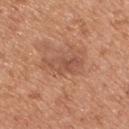<record>
  <biopsy_status>not biopsied; imaged during a skin examination</biopsy_status>
  <site>chest</site>
  <image>
    <source>total-body photography crop</source>
    <field_of_view_mm>15</field_of_view_mm>
  </image>
  <lighting>white-light</lighting>
  <lesion_size>
    <long_diameter_mm_approx>4.0</long_diameter_mm_approx>
  </lesion_size>
  <patient>
    <sex>male</sex>
    <age_approx>55</age_approx>
  </patient>
</record>